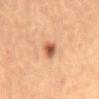This lesion was catalogued during total-body skin photography and was not selected for biopsy. A 15 mm close-up extracted from a 3D total-body photography capture. Longest diameter approximately 2.5 mm. Located on the back. Automated image analysis of the tile measured a classifier nevus-likeness of about 95/100. A male subject, aged around 55.